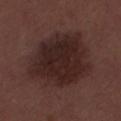No biopsy was performed on this lesion — it was imaged during a full skin examination and was not determined to be concerning. A male patient, aged 28–32. Approximately 8 mm at its widest. On the mid back. This is a white-light tile. A 15 mm close-up tile from a total-body photography series done for melanoma screening.